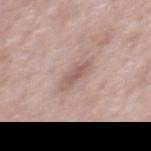Acquisition and patient details: The subject is a male aged around 55. Measured at roughly 3.5 mm in maximum diameter. The total-body-photography lesion software estimated a lesion area of about 4.5 mm². The analysis additionally found a border-irregularity rating of about 4/10, a within-lesion color-variation index near 1/10, and radial color variation of about 0.5. Imaged with white-light lighting. On the chest. A close-up tile cropped from a whole-body skin photograph, about 15 mm across.